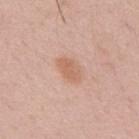Case summary:
* workup · no biopsy performed (imaged during a skin exam)
* patient · male, about 50 years old
* body site · the back
* image source · 15 mm crop, total-body photography